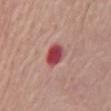Clinical impression: Part of a total-body skin-imaging series; this lesion was reviewed on a skin check and was not flagged for biopsy. Background: Approximately 3 mm at its widest. This image is a 15 mm lesion crop taken from a total-body photograph. Automated image analysis of the tile measured an average lesion color of about L≈47 a*≈35 b*≈22 (CIELAB). And it measured a border-irregularity index near 2/10, a color-variation rating of about 5/10, and peripheral color asymmetry of about 1.5. The analysis additionally found an automated nevus-likeness rating near 0 out of 100 and lesion-presence confidence of about 100/100. A male subject aged around 85 or older. From the chest. This is a white-light tile.